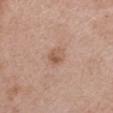notes = imaged on a skin check; not biopsied
image = ~15 mm tile from a whole-body skin photo
patient = female, approximately 45 years of age
body site = the right upper arm
lesion size = about 2.5 mm